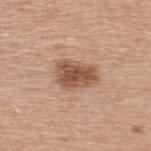Case summary:
- follow-up · total-body-photography surveillance lesion; no biopsy
- lesion diameter · ~4.5 mm (longest diameter)
- patient · male, aged around 45
- image · ~15 mm crop, total-body skin-cancer survey
- TBP lesion metrics · two-axis asymmetry of about 0.3; a mean CIELAB color near L≈53 a*≈21 b*≈30, roughly 13 lightness units darker than nearby skin, and a normalized lesion–skin contrast near 9
- tile lighting · white-light
- body site · the upper back